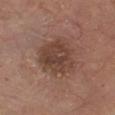This lesion was catalogued during total-body skin photography and was not selected for biopsy. A male subject, in their 80s. A 15 mm close-up tile from a total-body photography series done for melanoma screening. On the chest.